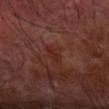follow-up = no biopsy performed (imaged during a skin exam); size = ~3 mm (longest diameter); subject = male, aged approximately 70; body site = the right forearm; illumination = cross-polarized; image source = 15 mm crop, total-body photography.